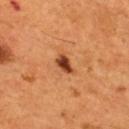<case>
<biopsy_status>not biopsied; imaged during a skin examination</biopsy_status>
<site>back</site>
<image>
  <source>total-body photography crop</source>
  <field_of_view_mm>15</field_of_view_mm>
</image>
<lighting>cross-polarized</lighting>
<patient>
  <sex>male</sex>
  <age_approx>55</age_approx>
</patient>
</case>